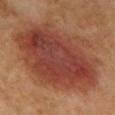Part of a total-body skin-imaging series; this lesion was reviewed on a skin check and was not flagged for biopsy. The total-body-photography lesion software estimated a normalized border contrast of about 9.5. The software also gave border irregularity of about 3 on a 0–10 scale, a within-lesion color-variation index near 5.5/10, and peripheral color asymmetry of about 1.5. It also reported a classifier nevus-likeness of about 100/100 and a lesion-detection confidence of about 100/100. Cropped from a whole-body photographic skin survey; the tile spans about 15 mm. Measured at roughly 12.5 mm in maximum diameter. Located on the chest. The tile uses cross-polarized illumination. A female subject, in their 60s.